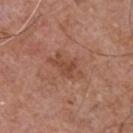Captured during whole-body skin photography for melanoma surveillance; the lesion was not biopsied.
Cropped from a total-body skin-imaging series; the visible field is about 15 mm.
Measured at roughly 4.5 mm in maximum diameter.
This is a white-light tile.
The lesion is on the chest.
A male patient, aged around 55.